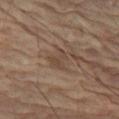Assessment: Captured during whole-body skin photography for melanoma surveillance; the lesion was not biopsied. Context: From the right leg. This is a cross-polarized tile. An algorithmic analysis of the crop reported an area of roughly 3.5 mm² and a shape-asymmetry score of about 0.6 (0 = symmetric). It also reported a border-irregularity index near 6.5/10, a within-lesion color-variation index near 0/10, and radial color variation of about 0. And it measured a nevus-likeness score of about 10/100 and lesion-presence confidence of about 90/100. The recorded lesion diameter is about 2.5 mm. A female patient roughly 80 years of age. A close-up tile cropped from a whole-body skin photograph, about 15 mm across.